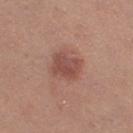Clinical impression:
This lesion was catalogued during total-body skin photography and was not selected for biopsy.
Clinical summary:
A region of skin cropped from a whole-body photographic capture, roughly 15 mm wide. This is a white-light tile. An algorithmic analysis of the crop reported border irregularity of about 2.5 on a 0–10 scale and a color-variation rating of about 3.5/10. And it measured a classifier nevus-likeness of about 65/100 and lesion-presence confidence of about 100/100. Longest diameter approximately 4 mm. A female patient aged around 40. On the leg.